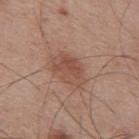| key | value |
|---|---|
| biopsy status | imaged on a skin check; not biopsied |
| imaging modality | 15 mm crop, total-body photography |
| anatomic site | the back |
| lighting | white-light illumination |
| diameter | ≈4.5 mm |
| patient | male, in their mid-50s |